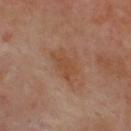<tbp_lesion>
  <site>upper back</site>
  <lighting>cross-polarized</lighting>
  <image>
    <source>total-body photography crop</source>
    <field_of_view_mm>15</field_of_view_mm>
  </image>
  <patient>
    <sex>male</sex>
    <age_approx>50</age_approx>
  </patient>
</tbp_lesion>